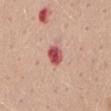Assessment:
The lesion was photographed on a routine skin check and not biopsied; there is no pathology result.
Context:
A region of skin cropped from a whole-body photographic capture, roughly 15 mm wide. The patient is a female aged 48–52. Located on the mid back. The tile uses white-light illumination. Automated image analysis of the tile measured border irregularity of about 1 on a 0–10 scale, a color-variation rating of about 4.5/10, and radial color variation of about 1. And it measured a classifier nevus-likeness of about 0/100 and a lesion-detection confidence of about 100/100.